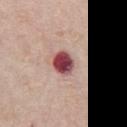{"biopsy_status": "not biopsied; imaged during a skin examination", "site": "chest", "image": {"source": "total-body photography crop", "field_of_view_mm": 15}, "patient": {"sex": "female", "age_approx": 65}}